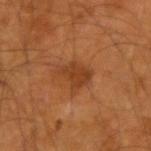Assessment:
Recorded during total-body skin imaging; not selected for excision or biopsy.
Clinical summary:
Longest diameter approximately 3.5 mm. A male patient, roughly 55 years of age. A roughly 15 mm field-of-view crop from a total-body skin photograph. Imaged with cross-polarized lighting. On the right forearm.